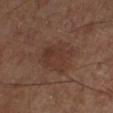Impression:
No biopsy was performed on this lesion — it was imaged during a full skin examination and was not determined to be concerning.
Image and clinical context:
The lesion-visualizer software estimated a mean CIELAB color near L≈34 a*≈18 b*≈24 and a normalized border contrast of about 5. And it measured a detector confidence of about 100 out of 100 that the crop contains a lesion. On the leg. A 15 mm crop from a total-body photograph taken for skin-cancer surveillance. A male patient, approximately 65 years of age. Approximately 5 mm at its widest. Captured under cross-polarized illumination.